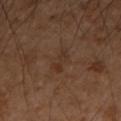  biopsy_status: not biopsied; imaged during a skin examination
  image:
    source: total-body photography crop
    field_of_view_mm: 15
  patient:
    sex: male
    age_approx: 55
  lesion_size:
    long_diameter_mm_approx: 3.0
  site: right forearm
  lighting: cross-polarized
  automated_metrics:
    cielab_L: 32
    cielab_a: 17
    cielab_b: 26
    vs_skin_darker_L: 5.0
    peripheral_color_asymmetry: 0.5